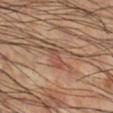workup: total-body-photography surveillance lesion; no biopsy
body site: the right lower leg
tile lighting: cross-polarized illumination
acquisition: ~15 mm crop, total-body skin-cancer survey
diameter: ≈3 mm
subject: male, roughly 60 years of age